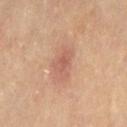Findings:
- workup: imaged on a skin check; not biopsied
- automated lesion analysis: a border-irregularity rating of about 4.5/10 and peripheral color asymmetry of about 1; lesion-presence confidence of about 100/100
- image source: ~15 mm tile from a whole-body skin photo
- site: the left thigh
- diameter: ≈3.5 mm
- subject: female, roughly 50 years of age